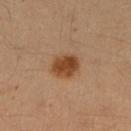Context:
Automated image analysis of the tile measured a lesion area of about 8 mm², an eccentricity of roughly 0.6, and a shape-asymmetry score of about 0.15 (0 = symmetric). It also reported a border-irregularity index near 1.5/10, a color-variation rating of about 3.5/10, and peripheral color asymmetry of about 1. The analysis additionally found an automated nevus-likeness rating near 100 out of 100 and lesion-presence confidence of about 100/100. The lesion is on the right upper arm. A female patient aged 33 to 37. Longest diameter approximately 3.5 mm. A 15 mm crop from a total-body photograph taken for skin-cancer surveillance.